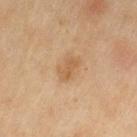  biopsy_status: not biopsied; imaged during a skin examination
  patient:
    sex: female
    age_approx: 60
  image:
    source: total-body photography crop
    field_of_view_mm: 15
  lighting: cross-polarized
  automated_metrics:
    border_irregularity_0_10: 2.5
    peripheral_color_asymmetry: 0.5
  lesion_size:
    long_diameter_mm_approx: 3.0
  site: leg